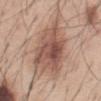No biopsy was performed on this lesion — it was imaged during a full skin examination and was not determined to be concerning.
Measured at roughly 8.5 mm in maximum diameter.
The subject is a male in their mid- to late 40s.
A 15 mm close-up extracted from a 3D total-body photography capture.
Automated image analysis of the tile measured a mean CIELAB color near L≈54 a*≈20 b*≈26 and roughly 12 lightness units darker than nearby skin. The analysis additionally found a border-irregularity rating of about 3.5/10, internal color variation of about 7 on a 0–10 scale, and peripheral color asymmetry of about 2.5.
On the abdomen.
Imaged with white-light lighting.